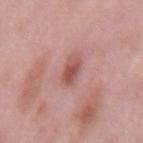Imaged with white-light lighting. Cropped from a total-body skin-imaging series; the visible field is about 15 mm. The lesion is on the mid back. Automated tile analysis of the lesion measured a footprint of about 5 mm², a shape eccentricity near 0.75, and a symmetry-axis asymmetry near 0.25. It also reported an average lesion color of about L≈53 a*≈25 b*≈25 (CIELAB) and a lesion–skin lightness drop of about 11. It also reported a within-lesion color-variation index near 4/10 and peripheral color asymmetry of about 1. About 3 mm across. A male patient in their mid- to late 50s.